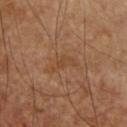workup — no biopsy performed (imaged during a skin exam) | location — the chest | acquisition — ~15 mm tile from a whole-body skin photo | automated metrics — a footprint of about 5 mm² and two-axis asymmetry of about 0.55 | patient — male, aged around 60 | tile lighting — cross-polarized illumination | lesion diameter — about 4 mm.